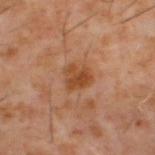Recorded during total-body skin imaging; not selected for excision or biopsy.
From the upper back.
An algorithmic analysis of the crop reported a lesion color around L≈43 a*≈23 b*≈35 in CIELAB and about 9 CIELAB-L* units darker than the surrounding skin.
This is a cross-polarized tile.
A 15 mm close-up extracted from a 3D total-body photography capture.
Longest diameter approximately 2.5 mm.
The patient is a male roughly 60 years of age.